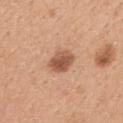workup — catalogued during a skin exam; not biopsied
automated lesion analysis — an automated nevus-likeness rating near 95 out of 100 and a detector confidence of about 100 out of 100 that the crop contains a lesion
acquisition — ~15 mm tile from a whole-body skin photo
location — the upper back
patient — female, aged 28 to 32